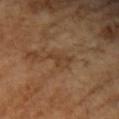| feature | finding |
|---|---|
| biopsy status | total-body-photography surveillance lesion; no biopsy |
| tile lighting | cross-polarized illumination |
| imaging modality | ~15 mm tile from a whole-body skin photo |
| anatomic site | the arm |
| diameter | about 3 mm |
| patient | female, roughly 70 years of age |